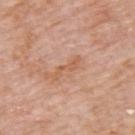Clinical impression:
This lesion was catalogued during total-body skin photography and was not selected for biopsy.
Acquisition and patient details:
Located on the upper back. A male subject, aged 73–77. This image is a 15 mm lesion crop taken from a total-body photograph. Imaged with white-light lighting.